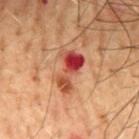Recorded during total-body skin imaging; not selected for excision or biopsy. The tile uses cross-polarized illumination. The subject is a male approximately 50 years of age. A region of skin cropped from a whole-body photographic capture, roughly 15 mm wide. The lesion is located on the mid back. Longest diameter approximately 4.5 mm.